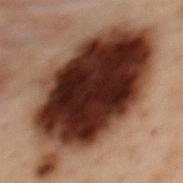Findings:
• follow-up — imaged on a skin check; not biopsied
• subject — female, aged 53–57
• anatomic site — the upper back
• image source — ~15 mm crop, total-body skin-cancer survey